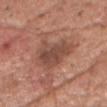- automated metrics: an outline eccentricity of about 0.8 (0 = round, 1 = elongated) and a symmetry-axis asymmetry near 0.35; a lesion color around L≈47 a*≈22 b*≈27 in CIELAB, roughly 10 lightness units darker than nearby skin, and a normalized border contrast of about 7.5
- size: ~5.5 mm (longest diameter)
- patient: male, approximately 80 years of age
- imaging modality: ~15 mm tile from a whole-body skin photo
- site: the chest
- tile lighting: white-light